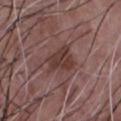A 15 mm close-up tile from a total-body photography series done for melanoma screening. The subject is a male roughly 50 years of age. The tile uses white-light illumination. From the front of the torso. About 4 mm across.